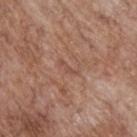<case>
  <biopsy_status>not biopsied; imaged during a skin examination</biopsy_status>
  <lighting>white-light</lighting>
  <site>back</site>
  <patient>
    <sex>male</sex>
    <age_approx>70</age_approx>
  </patient>
  <image>
    <source>total-body photography crop</source>
    <field_of_view_mm>15</field_of_view_mm>
  </image>
  <lesion_size>
    <long_diameter_mm_approx>2.5</long_diameter_mm_approx>
  </lesion_size>
  <automated_metrics>
    <area_mm2_approx>2.0</area_mm2_approx>
    <eccentricity>0.95</eccentricity>
    <vs_skin_darker_L>6.0</vs_skin_darker_L>
    <vs_skin_contrast_norm>4.5</vs_skin_contrast_norm>
    <border_irregularity_0_10>6.0</border_irregularity_0_10>
    <color_variation_0_10>0.0</color_variation_0_10>
    <peripheral_color_asymmetry>0.0</peripheral_color_asymmetry>
    <nevus_likeness_0_100>0</nevus_likeness_0_100>
  </automated_metrics>
</case>